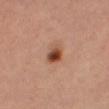The lesion was tiled from a total-body skin photograph and was not biopsied. The tile uses cross-polarized illumination. Measured at roughly 2.5 mm in maximum diameter. A lesion tile, about 15 mm wide, cut from a 3D total-body photograph. On the right thigh. The patient is a female roughly 45 years of age. Automated tile analysis of the lesion measured an outline eccentricity of about 0.65 (0 = round, 1 = elongated) and two-axis asymmetry of about 0.2. The software also gave a mean CIELAB color near L≈45 a*≈23 b*≈31, about 13 CIELAB-L* units darker than the surrounding skin, and a lesion-to-skin contrast of about 10 (normalized; higher = more distinct). It also reported a border-irregularity rating of about 2/10, internal color variation of about 8.5 on a 0–10 scale, and radial color variation of about 2.5.